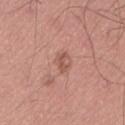Assessment:
Recorded during total-body skin imaging; not selected for excision or biopsy.
Acquisition and patient details:
This image is a 15 mm lesion crop taken from a total-body photograph. The patient is a male aged 48 to 52. On the lower back.